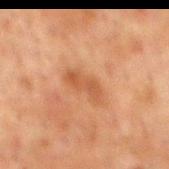Imaged during a routine full-body skin examination; the lesion was not biopsied and no histopathology is available. From the mid back. A male subject, approximately 60 years of age. Approximately 3.5 mm at its widest. A lesion tile, about 15 mm wide, cut from a 3D total-body photograph.